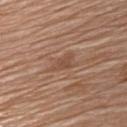Captured during whole-body skin photography for melanoma surveillance; the lesion was not biopsied. A male patient aged 78–82. The lesion is on the chest. Cropped from a total-body skin-imaging series; the visible field is about 15 mm. Longest diameter approximately 3.5 mm.